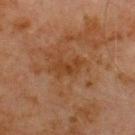Clinical impression: Part of a total-body skin-imaging series; this lesion was reviewed on a skin check and was not flagged for biopsy. Acquisition and patient details: A male subject aged 68–72. Located on the front of the torso. This image is a 15 mm lesion crop taken from a total-body photograph.